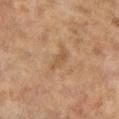{
  "biopsy_status": "not biopsied; imaged during a skin examination",
  "patient": {
    "sex": "female",
    "age_approx": 60
  },
  "site": "left lower leg",
  "image": {
    "source": "total-body photography crop",
    "field_of_view_mm": 15
  },
  "lighting": "cross-polarized"
}